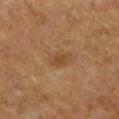Q: Was a biopsy performed?
A: no biopsy performed (imaged during a skin exam)
Q: What is the imaging modality?
A: ~15 mm tile from a whole-body skin photo
Q: Patient demographics?
A: male, aged 73–77
Q: What did automated image analysis measure?
A: a lesion area of about 4 mm² and two-axis asymmetry of about 0.2
Q: How was the tile lit?
A: cross-polarized
Q: Where on the body is the lesion?
A: the right thigh